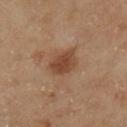<tbp_lesion>
<biopsy_status>not biopsied; imaged during a skin examination</biopsy_status>
<image>
  <source>total-body photography crop</source>
  <field_of_view_mm>15</field_of_view_mm>
</image>
<site>left lower leg</site>
<lesion_size>
  <long_diameter_mm_approx>3.5</long_diameter_mm_approx>
</lesion_size>
<patient>
  <sex>female</sex>
  <age_approx>60</age_approx>
</patient>
<lighting>cross-polarized</lighting>
</tbp_lesion>